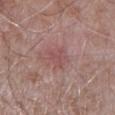No biopsy was performed on this lesion — it was imaged during a full skin examination and was not determined to be concerning. A region of skin cropped from a whole-body photographic capture, roughly 15 mm wide. The lesion is on the left upper arm. This is a white-light tile. A male patient, in their mid- to late 60s. Longest diameter approximately 2.5 mm.